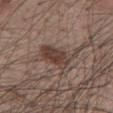{"biopsy_status": "not biopsied; imaged during a skin examination", "lighting": "white-light", "lesion_size": {"long_diameter_mm_approx": 4.5}, "automated_metrics": {"area_mm2_approx": 9.0, "shape_asymmetry": 0.25, "cielab_L": 40, "cielab_a": 16, "cielab_b": 23, "vs_skin_darker_L": 10.0, "vs_skin_contrast_norm": 8.5, "nevus_likeness_0_100": 95, "lesion_detection_confidence_0_100": 100}, "patient": {"sex": "male", "age_approx": 50}, "image": {"source": "total-body photography crop", "field_of_view_mm": 15}, "site": "mid back"}